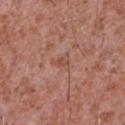Clinical impression: Recorded during total-body skin imaging; not selected for excision or biopsy. Background: The tile uses white-light illumination. A 15 mm close-up tile from a total-body photography series done for melanoma screening. Located on the chest. The subject is a male aged around 45. About 2.5 mm across. Automated tile analysis of the lesion measured a border-irregularity index near 3/10 and peripheral color asymmetry of about 0.5. And it measured an automated nevus-likeness rating near 0 out of 100.